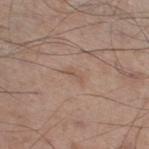body site = the right thigh
diameter = about 2.5 mm
lighting = white-light
imaging modality = total-body-photography crop, ~15 mm field of view
patient = male, approximately 60 years of age
TBP lesion metrics = border irregularity of about 4.5 on a 0–10 scale, a within-lesion color-variation index near 0/10, and a peripheral color-asymmetry measure near 0